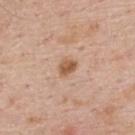This lesion was catalogued during total-body skin photography and was not selected for biopsy. A 15 mm close-up tile from a total-body photography series done for melanoma screening. The patient is a male approximately 60 years of age. From the back.